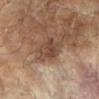Imaged during a routine full-body skin examination; the lesion was not biopsied and no histopathology is available.
A lesion tile, about 15 mm wide, cut from a 3D total-body photograph.
The lesion's longest dimension is about 4 mm.
On the arm.
A female subject aged around 80.